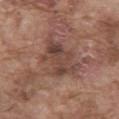patient:
  sex: male
  age_approx: 75
site: abdomen
image:
  source: total-body photography crop
  field_of_view_mm: 15